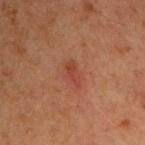notes: imaged on a skin check; not biopsied
site: the chest
subject: male, aged 58–62
illumination: cross-polarized illumination
diameter: about 3 mm
image source: 15 mm crop, total-body photography
automated metrics: a mean CIELAB color near L≈33 a*≈22 b*≈25 and a lesion-to-skin contrast of about 5.5 (normalized; higher = more distinct); border irregularity of about 3.5 on a 0–10 scale and a peripheral color-asymmetry measure near 0.5; a classifier nevus-likeness of about 10/100 and lesion-presence confidence of about 100/100